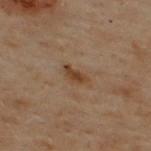Assessment: Captured during whole-body skin photography for melanoma surveillance; the lesion was not biopsied. Clinical summary: From the upper back. The patient is a male aged around 50. A 15 mm crop from a total-body photograph taken for skin-cancer surveillance. The recorded lesion diameter is about 3 mm. Imaged with cross-polarized lighting.